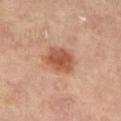notes: imaged on a skin check; not biopsied
image source: total-body-photography crop, ~15 mm field of view
tile lighting: cross-polarized
lesion size: about 3.5 mm
site: the left leg
subject: female, aged 63 to 67